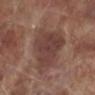Q: Is there a histopathology result?
A: imaged on a skin check; not biopsied
Q: How large is the lesion?
A: ≈9.5 mm
Q: Patient demographics?
A: male, aged approximately 70
Q: Where on the body is the lesion?
A: the leg
Q: How was the tile lit?
A: white-light
Q: What did automated image analysis measure?
A: a footprint of about 36 mm² and two-axis asymmetry of about 0.25; about 8 CIELAB-L* units darker than the surrounding skin and a normalized border contrast of about 6.5; an automated nevus-likeness rating near 5 out of 100 and lesion-presence confidence of about 100/100
Q: What kind of image is this?
A: ~15 mm crop, total-body skin-cancer survey